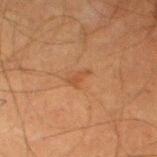follow-up: catalogued during a skin exam; not biopsied
image: 15 mm crop, total-body photography
subject: male, aged around 60
diameter: ≈2.5 mm
location: the left thigh
illumination: cross-polarized